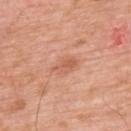| feature | finding |
|---|---|
| biopsy status | imaged on a skin check; not biopsied |
| patient | male, roughly 60 years of age |
| acquisition | ~15 mm crop, total-body skin-cancer survey |
| body site | the upper back |